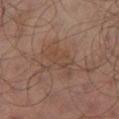biopsy status: catalogued during a skin exam; not biopsied | patient: male, roughly 65 years of age | lesion diameter: ~4.5 mm (longest diameter) | location: the left lower leg | tile lighting: cross-polarized illumination | imaging modality: ~15 mm tile from a whole-body skin photo.